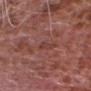Imaged during a routine full-body skin examination; the lesion was not biopsied and no histopathology is available.